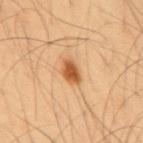Part of a total-body skin-imaging series; this lesion was reviewed on a skin check and was not flagged for biopsy.
The lesion is on the mid back.
The tile uses cross-polarized illumination.
Approximately 3.5 mm at its widest.
The lesion-visualizer software estimated a border-irregularity rating of about 3/10, a within-lesion color-variation index near 2.5/10, and peripheral color asymmetry of about 0.5. The software also gave lesion-presence confidence of about 100/100.
A 15 mm close-up extracted from a 3D total-body photography capture.
The patient is a male aged around 55.